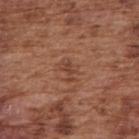Recorded during total-body skin imaging; not selected for excision or biopsy. A close-up tile cropped from a whole-body skin photograph, about 15 mm across. Longest diameter approximately 3 mm. A male patient, roughly 75 years of age. Captured under white-light illumination. On the right upper arm.